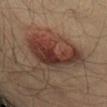| field | value |
|---|---|
| follow-up | catalogued during a skin exam; not biopsied |
| diameter | ~7.5 mm (longest diameter) |
| TBP lesion metrics | a mean CIELAB color near L≈36 a*≈20 b*≈24 and a lesion-to-skin contrast of about 11 (normalized; higher = more distinct); a border-irregularity index near 6/10 and a within-lesion color-variation index near 7/10 |
| site | the front of the torso |
| image | 15 mm crop, total-body photography |
| illumination | cross-polarized |
| subject | male, aged around 40 |